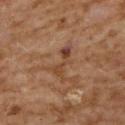Part of a total-body skin-imaging series; this lesion was reviewed on a skin check and was not flagged for biopsy.
Automated image analysis of the tile measured a footprint of about 5 mm², a shape eccentricity near 0.95, and a shape-asymmetry score of about 0.55 (0 = symmetric).
The recorded lesion diameter is about 4.5 mm.
A 15 mm close-up tile from a total-body photography series done for melanoma screening.
A female patient aged around 60.
The tile uses cross-polarized illumination.
On the upper back.